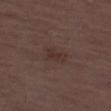biopsy status — total-body-photography surveillance lesion; no biopsy | subject — male, approximately 70 years of age | location — the left thigh | automated metrics — about 5 CIELAB-L* units darker than the surrounding skin and a normalized lesion–skin contrast near 6.5 | image source — total-body-photography crop, ~15 mm field of view.